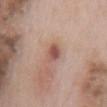<record>
  <biopsy_status>not biopsied; imaged during a skin examination</biopsy_status>
  <image>
    <source>total-body photography crop</source>
    <field_of_view_mm>15</field_of_view_mm>
  </image>
  <lighting>white-light</lighting>
  <lesion_size>
    <long_diameter_mm_approx>2.5</long_diameter_mm_approx>
  </lesion_size>
  <automated_metrics>
    <cielab_L>53</cielab_L>
    <cielab_a>23</cielab_a>
    <cielab_b>25</cielab_b>
    <vs_skin_darker_L>12.0</vs_skin_darker_L>
    <vs_skin_contrast_norm>8.0</vs_skin_contrast_norm>
    <lesion_detection_confidence_0_100>100</lesion_detection_confidence_0_100>
  </automated_metrics>
  <patient>
    <sex>male</sex>
    <age_approx>65</age_approx>
  </patient>
  <site>mid back</site>
</record>